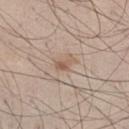Captured during whole-body skin photography for melanoma surveillance; the lesion was not biopsied.
The lesion-visualizer software estimated an average lesion color of about L≈57 a*≈16 b*≈28 (CIELAB), roughly 8 lightness units darker than nearby skin, and a normalized lesion–skin contrast near 6.5.
The lesion is on the left thigh.
A male subject, roughly 45 years of age.
Captured under white-light illumination.
A roughly 15 mm field-of-view crop from a total-body skin photograph.
About 2.5 mm across.